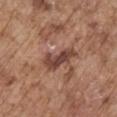biopsy status: imaged on a skin check; not biopsied | lighting: white-light | lesion diameter: ≈4.5 mm | anatomic site: the right upper arm | image: ~15 mm crop, total-body skin-cancer survey | patient: male, aged 73–77.